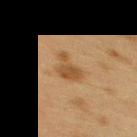Clinical impression:
Captured during whole-body skin photography for melanoma surveillance; the lesion was not biopsied.
Image and clinical context:
This is a cross-polarized tile. Automated tile analysis of the lesion measured an area of roughly 6 mm², an eccentricity of roughly 0.5, and a symmetry-axis asymmetry near 0.55. The software also gave radial color variation of about 0.5. The software also gave a classifier nevus-likeness of about 20/100 and a lesion-detection confidence of about 100/100. The lesion's longest dimension is about 3 mm. A roughly 15 mm field-of-view crop from a total-body skin photograph. The lesion is located on the upper back. The subject is a female aged 38–42.